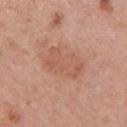Acquisition and patient details:
From the right upper arm. A female patient, in their mid- to late 50s. A lesion tile, about 15 mm wide, cut from a 3D total-body photograph. About 5.5 mm across. This is a white-light tile. The total-body-photography lesion software estimated an area of roughly 15 mm², an eccentricity of roughly 0.85, and a shape-asymmetry score of about 0.2 (0 = symmetric). It also reported a lesion color around L≈57 a*≈23 b*≈30 in CIELAB, roughly 7 lightness units darker than nearby skin, and a normalized border contrast of about 5. The analysis additionally found border irregularity of about 3 on a 0–10 scale, internal color variation of about 2.5 on a 0–10 scale, and peripheral color asymmetry of about 1. The software also gave an automated nevus-likeness rating near 10 out of 100 and a lesion-detection confidence of about 100/100.